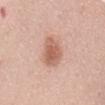Assessment: The lesion was photographed on a routine skin check and not biopsied; there is no pathology result. Clinical summary: From the mid back. The recorded lesion diameter is about 4.5 mm. Cropped from a whole-body photographic skin survey; the tile spans about 15 mm. The subject is a female aged 63–67. This is a white-light tile.